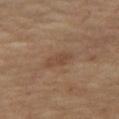Q: Is there a histopathology result?
A: imaged on a skin check; not biopsied
Q: Who is the patient?
A: female
Q: What is the imaging modality?
A: ~15 mm crop, total-body skin-cancer survey
Q: Where on the body is the lesion?
A: the leg